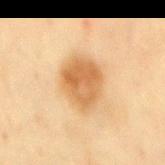The lesion was photographed on a routine skin check and not biopsied; there is no pathology result. A 15 mm crop from a total-body photograph taken for skin-cancer surveillance. Captured under cross-polarized illumination. About 5.5 mm across. A male patient approximately 45 years of age. On the mid back. Automated tile analysis of the lesion measured a mean CIELAB color near L≈55 a*≈19 b*≈38 and a lesion–skin lightness drop of about 11. The software also gave a detector confidence of about 100 out of 100 that the crop contains a lesion.